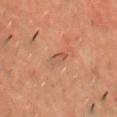Impression:
No biopsy was performed on this lesion — it was imaged during a full skin examination and was not determined to be concerning.
Acquisition and patient details:
The lesion is on the chest. Longest diameter approximately 3 mm. A male subject aged 48–52. A roughly 15 mm field-of-view crop from a total-body skin photograph. The total-body-photography lesion software estimated an average lesion color of about L≈41 a*≈19 b*≈25 (CIELAB), about 6 CIELAB-L* units darker than the surrounding skin, and a normalized border contrast of about 5. The software also gave internal color variation of about 0 on a 0–10 scale and radial color variation of about 0. This is a cross-polarized tile.